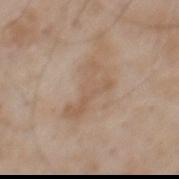Impression:
Captured during whole-body skin photography for melanoma surveillance; the lesion was not biopsied.
Background:
The subject is a male aged around 50. Located on the mid back. An algorithmic analysis of the crop reported a lesion color around L≈57 a*≈15 b*≈29 in CIELAB, about 7 CIELAB-L* units darker than the surrounding skin, and a normalized lesion–skin contrast near 5. The software also gave a detector confidence of about 100 out of 100 that the crop contains a lesion. About 6 mm across. A roughly 15 mm field-of-view crop from a total-body skin photograph. Captured under white-light illumination.